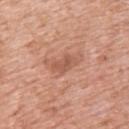A female patient, aged approximately 50. The lesion is on the upper back. The recorded lesion diameter is about 3 mm. The tile uses white-light illumination. This image is a 15 mm lesion crop taken from a total-body photograph.